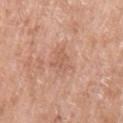Captured during whole-body skin photography for melanoma surveillance; the lesion was not biopsied. An algorithmic analysis of the crop reported a detector confidence of about 100 out of 100 that the crop contains a lesion. The recorded lesion diameter is about 3 mm. A female subject, roughly 60 years of age. A 15 mm crop from a total-body photograph taken for skin-cancer surveillance. Imaged with white-light lighting. On the left upper arm.